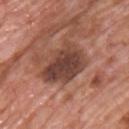  biopsy_status: not biopsied; imaged during a skin examination
  lighting: white-light
  automated_metrics:
    cielab_L: 42
    cielab_a: 22
    cielab_b: 26
    vs_skin_darker_L: 13.0
    vs_skin_contrast_norm: 10.0
    border_irregularity_0_10: 3.0
    color_variation_0_10: 5.0
    peripheral_color_asymmetry: 1.5
  site: upper back
  image:
    source: total-body photography crop
    field_of_view_mm: 15
  patient:
    sex: male
    age_approx: 75
  lesion_size:
    long_diameter_mm_approx: 6.0